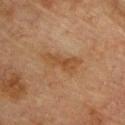Recorded during total-body skin imaging; not selected for excision or biopsy.
Approximately 5 mm at its widest.
This is a cross-polarized tile.
A lesion tile, about 15 mm wide, cut from a 3D total-body photograph.
The subject is a male roughly 75 years of age.
The lesion is located on the chest.